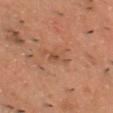Clinical impression: The lesion was photographed on a routine skin check and not biopsied; there is no pathology result. Context: Automated image analysis of the tile measured an area of roughly 3.5 mm², an outline eccentricity of about 0.85 (0 = round, 1 = elongated), and two-axis asymmetry of about 0.35. The analysis additionally found an average lesion color of about L≈38 a*≈18 b*≈26 (CIELAB), a lesion–skin lightness drop of about 5, and a lesion-to-skin contrast of about 5 (normalized; higher = more distinct). The software also gave border irregularity of about 3 on a 0–10 scale, a color-variation rating of about 3.5/10, and radial color variation of about 1. The analysis additionally found a nevus-likeness score of about 0/100. The lesion is on the chest. The patient is a male approximately 50 years of age. A 15 mm crop from a total-body photograph taken for skin-cancer surveillance. Imaged with cross-polarized lighting. The recorded lesion diameter is about 3 mm.